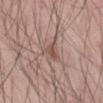This lesion was catalogued during total-body skin photography and was not selected for biopsy.
A male subject aged approximately 45.
Located on the back.
A 15 mm close-up tile from a total-body photography series done for melanoma screening.
Captured under white-light illumination.
The recorded lesion diameter is about 3 mm.